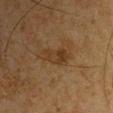Part of a total-body skin-imaging series; this lesion was reviewed on a skin check and was not flagged for biopsy. A male patient, in their mid- to late 60s. The recorded lesion diameter is about 3.5 mm. Located on the arm. This image is a 15 mm lesion crop taken from a total-body photograph.